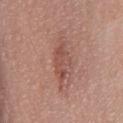biopsy status — total-body-photography surveillance lesion; no biopsy
acquisition — total-body-photography crop, ~15 mm field of view
tile lighting — white-light illumination
size — ~5.5 mm (longest diameter)
subject — male, aged approximately 55
site — the chest